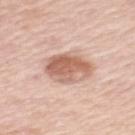Findings:
• workup · imaged on a skin check; not biopsied
• location · the left upper arm
• size · about 5.5 mm
• imaging modality · ~15 mm crop, total-body skin-cancer survey
• illumination · white-light illumination
• patient · female, approximately 50 years of age